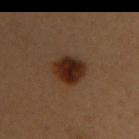A 15 mm crop from a total-body photograph taken for skin-cancer surveillance.
This is a cross-polarized tile.
Located on the left upper arm.
A female patient, aged 48–52.